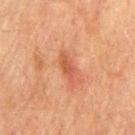Imaged during a routine full-body skin examination; the lesion was not biopsied and no histopathology is available.
This image is a 15 mm lesion crop taken from a total-body photograph.
Located on the chest.
A male subject in their mid- to late 70s.